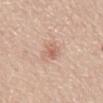Q: Was this lesion biopsied?
A: no biopsy performed (imaged during a skin exam)
Q: Where on the body is the lesion?
A: the mid back
Q: Patient demographics?
A: male, aged around 60
Q: What did automated image analysis measure?
A: a nevus-likeness score of about 10/100 and lesion-presence confidence of about 100/100
Q: Lesion size?
A: ≈2.5 mm
Q: What lighting was used for the tile?
A: white-light
Q: How was this image acquired?
A: total-body-photography crop, ~15 mm field of view